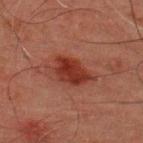follow-up=imaged on a skin check; not biopsied | image source=~15 mm crop, total-body skin-cancer survey | patient=male, roughly 45 years of age | tile lighting=cross-polarized | body site=the upper back.